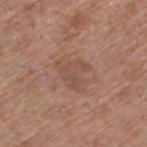{
  "biopsy_status": "not biopsied; imaged during a skin examination",
  "lighting": "white-light",
  "site": "left thigh",
  "automated_metrics": {
    "area_mm2_approx": 9.5,
    "eccentricity": 0.6,
    "shape_asymmetry": 0.45,
    "nevus_likeness_0_100": 0,
    "lesion_detection_confidence_0_100": 100
  },
  "lesion_size": {
    "long_diameter_mm_approx": 4.0
  },
  "patient": {
    "sex": "female",
    "age_approx": 75
  },
  "image": {
    "source": "total-body photography crop",
    "field_of_view_mm": 15
  }
}